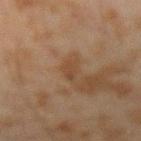Imaged during a routine full-body skin examination; the lesion was not biopsied and no histopathology is available. This image is a 15 mm lesion crop taken from a total-body photograph. The subject is a male aged approximately 45. From the arm. Measured at roughly 3 mm in maximum diameter. Automated image analysis of the tile measured a mean CIELAB color near L≈36 a*≈15 b*≈26, about 6 CIELAB-L* units darker than the surrounding skin, and a lesion-to-skin contrast of about 5.5 (normalized; higher = more distinct). The software also gave a border-irregularity index near 3/10, a within-lesion color-variation index near 1/10, and a peripheral color-asymmetry measure near 0. The tile uses cross-polarized illumination.